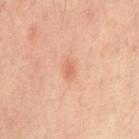Recorded during total-body skin imaging; not selected for excision or biopsy.
The tile uses cross-polarized illumination.
Measured at roughly 1.5 mm in maximum diameter.
A 15 mm close-up tile from a total-body photography series done for melanoma screening.
A male subject aged approximately 65.
From the front of the torso.
Automated tile analysis of the lesion measured an area of roughly 1 mm², an eccentricity of roughly 0.75, and a symmetry-axis asymmetry near 0.3. And it measured a lesion color around L≈61 a*≈27 b*≈33 in CIELAB, roughly 8 lightness units darker than nearby skin, and a normalized lesion–skin contrast near 5.5. And it measured a border-irregularity index near 2/10, a color-variation rating of about 0/10, and a peripheral color-asymmetry measure near 0. And it measured an automated nevus-likeness rating near 40 out of 100 and a detector confidence of about 100 out of 100 that the crop contains a lesion.